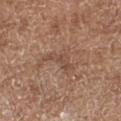Case summary:
• biopsy status — total-body-photography surveillance lesion; no biopsy
• body site — the left forearm
• automated lesion analysis — a footprint of about 4.5 mm² and a shape eccentricity near 0.9; a mean CIELAB color near L≈48 a*≈19 b*≈28 and a normalized border contrast of about 5.5; a border-irregularity rating of about 7/10, a color-variation rating of about 1/10, and radial color variation of about 0; lesion-presence confidence of about 85/100
• size — about 4 mm
• imaging modality — 15 mm crop, total-body photography
• subject — female, aged approximately 75